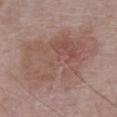Part of a total-body skin-imaging series; this lesion was reviewed on a skin check and was not flagged for biopsy. Imaged with white-light lighting. The recorded lesion diameter is about 10.5 mm. A male subject in their mid- to late 80s or older. A lesion tile, about 15 mm wide, cut from a 3D total-body photograph. The lesion is located on the chest.